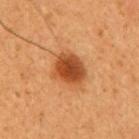notes: total-body-photography surveillance lesion; no biopsy
anatomic site: the mid back
tile lighting: cross-polarized
image: ~15 mm crop, total-body skin-cancer survey
patient: male, aged 43–47